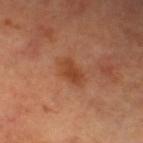This image is a 15 mm lesion crop taken from a total-body photograph.
Longest diameter approximately 3.5 mm.
Automated image analysis of the tile measured a mean CIELAB color near L≈46 a*≈27 b*≈36, about 8 CIELAB-L* units darker than the surrounding skin, and a normalized lesion–skin contrast near 7. It also reported a nevus-likeness score of about 65/100 and a detector confidence of about 100 out of 100 that the crop contains a lesion.
From the left lower leg.
The patient is a female aged around 60.